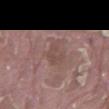Assessment: Part of a total-body skin-imaging series; this lesion was reviewed on a skin check and was not flagged for biopsy. Background: The total-body-photography lesion software estimated a border-irregularity index near 5.5/10, a color-variation rating of about 0/10, and radial color variation of about 0. The analysis additionally found a nevus-likeness score of about 0/100 and lesion-presence confidence of about 80/100. Imaged with white-light lighting. A region of skin cropped from a whole-body photographic capture, roughly 15 mm wide. The patient is a male in their 40s. Longest diameter approximately 3.5 mm. Located on the back.